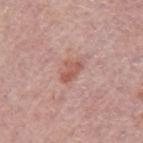The lesion was tiled from a total-body skin photograph and was not biopsied. Located on the left upper arm. A female subject, about 65 years old. A close-up tile cropped from a whole-body skin photograph, about 15 mm across.